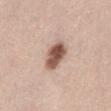A close-up tile cropped from a whole-body skin photograph, about 15 mm across.
The total-body-photography lesion software estimated a footprint of about 8 mm², an outline eccentricity of about 0.7 (0 = round, 1 = elongated), and a shape-asymmetry score of about 0.15 (0 = symmetric). The analysis additionally found border irregularity of about 1.5 on a 0–10 scale, a color-variation rating of about 5/10, and radial color variation of about 2. And it measured an automated nevus-likeness rating near 95 out of 100 and a lesion-detection confidence of about 100/100.
Captured under white-light illumination.
The subject is a male aged 43 to 47.
The lesion is located on the left thigh.